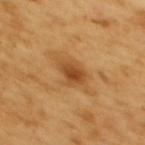{
  "biopsy_status": "not biopsied; imaged during a skin examination",
  "lighting": "cross-polarized",
  "lesion_size": {
    "long_diameter_mm_approx": 3.0
  },
  "automated_metrics": {
    "cielab_L": 48,
    "cielab_a": 24,
    "cielab_b": 42,
    "vs_skin_darker_L": 11.0,
    "vs_skin_contrast_norm": 8.0
  },
  "patient": {
    "sex": "female",
    "age_approx": 55
  },
  "site": "back",
  "image": {
    "source": "total-body photography crop",
    "field_of_view_mm": 15
  }
}